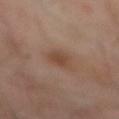notes — catalogued during a skin exam; not biopsied
subject — male, aged 48 to 52
image — ~15 mm crop, total-body skin-cancer survey
tile lighting — cross-polarized illumination
image-analysis metrics — a lesion color around L≈44 a*≈18 b*≈28 in CIELAB and a lesion-to-skin contrast of about 6.5 (normalized; higher = more distinct); a border-irregularity index near 2/10
lesion size — ~3 mm (longest diameter)
anatomic site — the back